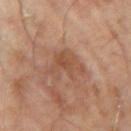Clinical impression:
Imaged during a routine full-body skin examination; the lesion was not biopsied and no histopathology is available.
Context:
Cropped from a total-body skin-imaging series; the visible field is about 15 mm. Automated tile analysis of the lesion measured a lesion color around L≈48 a*≈20 b*≈30 in CIELAB, about 7 CIELAB-L* units darker than the surrounding skin, and a normalized border contrast of about 5.5. The software also gave a border-irregularity rating of about 6.5/10 and a color-variation rating of about 3.5/10. The software also gave an automated nevus-likeness rating near 0 out of 100. The lesion is located on the right upper arm. Imaged with cross-polarized lighting. Longest diameter approximately 4.5 mm. The subject is a male in their mid-60s.